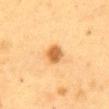workup — catalogued during a skin exam; not biopsied | patient — female, about 55 years old | tile lighting — cross-polarized | image source — ~15 mm tile from a whole-body skin photo | automated lesion analysis — an average lesion color of about L≈52 a*≈20 b*≈40 (CIELAB) and a normalized border contrast of about 9 | lesion diameter — about 2.5 mm | body site — the chest.